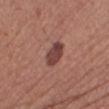Clinical impression:
Imaged during a routine full-body skin examination; the lesion was not biopsied and no histopathology is available.
Background:
The recorded lesion diameter is about 3.5 mm. Cropped from a total-body skin-imaging series; the visible field is about 15 mm. On the right lower leg. A female subject, approximately 40 years of age. This is a white-light tile.